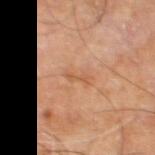• notes · imaged on a skin check; not biopsied
• body site · the right leg
• lighting · cross-polarized
• image · ~15 mm tile from a whole-body skin photo
• diameter · about 2.5 mm
• patient · male, about 60 years old
• TBP lesion metrics · a lesion area of about 2 mm² and a shape-asymmetry score of about 0.45 (0 = symmetric); a lesion color around L≈54 a*≈22 b*≈35 in CIELAB and roughly 7 lightness units darker than nearby skin; internal color variation of about 0 on a 0–10 scale and radial color variation of about 0; a nevus-likeness score of about 0/100